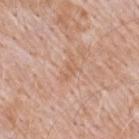{"biopsy_status": "not biopsied; imaged during a skin examination", "site": "back", "patient": {"sex": "male", "age_approx": 50}, "automated_metrics": {"area_mm2_approx": 4.0, "eccentricity": 0.85, "shape_asymmetry": 0.55}, "image": {"source": "total-body photography crop", "field_of_view_mm": 15}, "lighting": "white-light"}